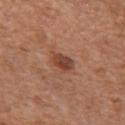{"biopsy_status": "not biopsied; imaged during a skin examination", "automated_metrics": {"eccentricity": 0.7, "cielab_L": 43, "cielab_a": 24, "cielab_b": 30, "vs_skin_darker_L": 11.0, "border_irregularity_0_10": 1.5, "color_variation_0_10": 5.5, "peripheral_color_asymmetry": 2.0, "nevus_likeness_0_100": 85, "lesion_detection_confidence_0_100": 100}, "site": "chest", "patient": {"sex": "female", "age_approx": 75}, "lighting": "white-light", "lesion_size": {"long_diameter_mm_approx": 3.0}, "image": {"source": "total-body photography crop", "field_of_view_mm": 15}}